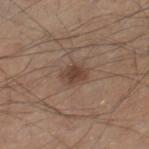biopsy_status: not biopsied; imaged during a skin examination
site: leg
lighting: white-light
lesion_size:
  long_diameter_mm_approx: 3.0
image:
  source: total-body photography crop
  field_of_view_mm: 15
patient:
  sex: male
  age_approx: 45